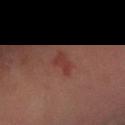| field | value |
|---|---|
| TBP lesion metrics | a shape-asymmetry score of about 0.45 (0 = symmetric); an average lesion color of about L≈35 a*≈25 b*≈23 (CIELAB) |
| image source | ~15 mm crop, total-body skin-cancer survey |
| illumination | cross-polarized illumination |
| subject | male, approximately 65 years of age |
| location | the left forearm |